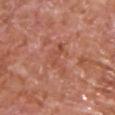| field | value |
|---|---|
| workup | no biopsy performed (imaged during a skin exam) |
| location | the chest |
| subject | male, aged 63 to 67 |
| acquisition | ~15 mm tile from a whole-body skin photo |